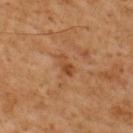follow-up = total-body-photography surveillance lesion; no biopsy | lesion diameter = about 2.5 mm | subject = male, in their 60s | body site = the upper back | acquisition = 15 mm crop, total-body photography | illumination = cross-polarized illumination | automated lesion analysis = a footprint of about 3 mm², an eccentricity of roughly 0.85, and two-axis asymmetry of about 0.35; an average lesion color of about L≈42 a*≈22 b*≈35 (CIELAB), about 8 CIELAB-L* units darker than the surrounding skin, and a normalized border contrast of about 6.5.